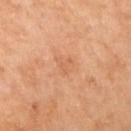{
  "biopsy_status": "not biopsied; imaged during a skin examination",
  "site": "right upper arm",
  "image": {
    "source": "total-body photography crop",
    "field_of_view_mm": 15
  },
  "patient": {
    "sex": "female",
    "age_approx": 65
  }
}